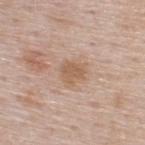workup — catalogued during a skin exam; not biopsied | image source — ~15 mm tile from a whole-body skin photo | body site — the upper back | patient — male, aged approximately 60.